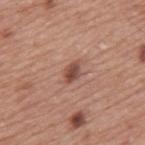  biopsy_status: not biopsied; imaged during a skin examination
  lesion_size:
    long_diameter_mm_approx: 3.0
  image:
    source: total-body photography crop
    field_of_view_mm: 15
  lighting: white-light
  patient:
    sex: male
    age_approx: 75
  site: mid back